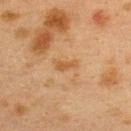- biopsy status — catalogued during a skin exam; not biopsied
- tile lighting — cross-polarized illumination
- size — ~3 mm (longest diameter)
- image — total-body-photography crop, ~15 mm field of view
- image-analysis metrics — a nevus-likeness score of about 0/100 and a lesion-detection confidence of about 100/100
- subject — female, roughly 40 years of age
- location — the back